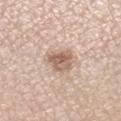The lesion was photographed on a routine skin check and not biopsied; there is no pathology result.
On the right lower leg.
A 15 mm close-up tile from a total-body photography series done for melanoma screening.
The subject is a female aged around 60.